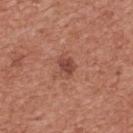| feature | finding |
|---|---|
| notes | total-body-photography surveillance lesion; no biopsy |
| patient | male, in their mid- to late 40s |
| TBP lesion metrics | a footprint of about 4 mm², an outline eccentricity of about 0.65 (0 = round, 1 = elongated), and two-axis asymmetry of about 0.25; an average lesion color of about L≈46 a*≈25 b*≈28 (CIELAB), about 10 CIELAB-L* units darker than the surrounding skin, and a normalized lesion–skin contrast near 7.5; border irregularity of about 2.5 on a 0–10 scale and internal color variation of about 2 on a 0–10 scale |
| image source | ~15 mm tile from a whole-body skin photo |
| diameter | about 2.5 mm |
| anatomic site | the chest |
| lighting | white-light |